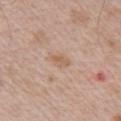follow-up: no biopsy performed (imaged during a skin exam)
tile lighting: white-light illumination
subject: male, in their mid- to late 60s
lesion size: ≈2.5 mm
TBP lesion metrics: a lesion area of about 3 mm² and an eccentricity of roughly 0.85; a lesion–skin lightness drop of about 8 and a lesion-to-skin contrast of about 6 (normalized; higher = more distinct); a classifier nevus-likeness of about 0/100 and a lesion-detection confidence of about 100/100
image: ~15 mm tile from a whole-body skin photo
anatomic site: the chest